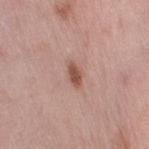Case summary:
- biopsy status: no biopsy performed (imaged during a skin exam)
- body site: the right thigh
- image: total-body-photography crop, ~15 mm field of view
- size: ≈3 mm
- tile lighting: white-light illumination
- patient: female, aged around 50